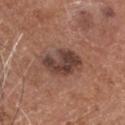Assessment: Part of a total-body skin-imaging series; this lesion was reviewed on a skin check and was not flagged for biopsy. Background: Cropped from a total-body skin-imaging series; the visible field is about 15 mm. A male subject aged approximately 60. The tile uses white-light illumination. Automated tile analysis of the lesion measured a lesion area of about 12 mm², an outline eccentricity of about 0.8 (0 = round, 1 = elongated), and two-axis asymmetry of about 0.25. The analysis additionally found peripheral color asymmetry of about 1.5. On the head or neck.